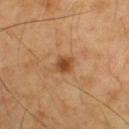Q: Is there a histopathology result?
A: imaged on a skin check; not biopsied
Q: What is the imaging modality?
A: total-body-photography crop, ~15 mm field of view
Q: Patient demographics?
A: male, aged around 55
Q: Where on the body is the lesion?
A: the back